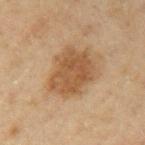{"biopsy_status": "not biopsied; imaged during a skin examination", "patient": {"sex": "male", "age_approx": 45}, "image": {"source": "total-body photography crop", "field_of_view_mm": 15}, "lighting": "cross-polarized", "lesion_size": {"long_diameter_mm_approx": 6.0}, "site": "left upper arm"}